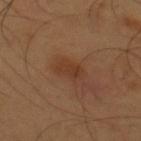The lesion was tiled from a total-body skin photograph and was not biopsied.
Located on the upper back.
The patient is a male roughly 55 years of age.
An algorithmic analysis of the crop reported a footprint of about 4.5 mm², a shape eccentricity near 0.8, and a symmetry-axis asymmetry near 0.25. The analysis additionally found a normalized border contrast of about 6. The analysis additionally found a border-irregularity index near 2.5/10 and a within-lesion color-variation index near 2/10.
A 15 mm crop from a total-body photograph taken for skin-cancer surveillance.
The lesion's longest dimension is about 2.5 mm.